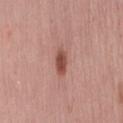Captured during whole-body skin photography for melanoma surveillance; the lesion was not biopsied. Imaged with white-light lighting. A female patient, approximately 40 years of age. A lesion tile, about 15 mm wide, cut from a 3D total-body photograph. The lesion is on the lower back. Measured at roughly 3 mm in maximum diameter. The total-body-photography lesion software estimated an area of roughly 4.5 mm², an eccentricity of roughly 0.8, and a symmetry-axis asymmetry near 0.2. The analysis additionally found roughly 13 lightness units darker than nearby skin and a normalized border contrast of about 9. The software also gave a border-irregularity rating of about 2/10, internal color variation of about 2.5 on a 0–10 scale, and radial color variation of about 1. And it measured a nevus-likeness score of about 95/100 and lesion-presence confidence of about 100/100.